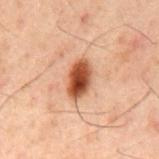Q: Was this lesion biopsied?
A: catalogued during a skin exam; not biopsied
Q: What are the patient's age and sex?
A: male, in their 60s
Q: What is the anatomic site?
A: the mid back
Q: What is the imaging modality?
A: ~15 mm tile from a whole-body skin photo